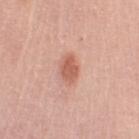Case summary:
• biopsy status — no biopsy performed (imaged during a skin exam)
• size — about 3 mm
• illumination — white-light
• body site — the left upper arm
• subject — female, aged 63–67
• acquisition — ~15 mm tile from a whole-body skin photo